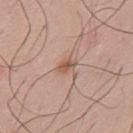The lesion was tiled from a total-body skin photograph and was not biopsied. The lesion is located on the mid back. A 15 mm close-up tile from a total-body photography series done for melanoma screening. A male patient aged 38 to 42. About 2.5 mm across. Imaged with white-light lighting. The total-body-photography lesion software estimated an area of roughly 3.5 mm², a shape eccentricity near 0.8, and a symmetry-axis asymmetry near 0.25. And it measured an average lesion color of about L≈56 a*≈19 b*≈28 (CIELAB), a lesion–skin lightness drop of about 10, and a normalized lesion–skin contrast near 7. And it measured a border-irregularity rating of about 2.5/10, internal color variation of about 4 on a 0–10 scale, and a peripheral color-asymmetry measure near 1.